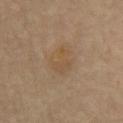Image and clinical context: A 15 mm close-up tile from a total-body photography series done for melanoma screening. Captured under cross-polarized illumination. Located on the front of the torso. The total-body-photography lesion software estimated a footprint of about 8.5 mm², a shape eccentricity near 0.8, and two-axis asymmetry of about 0.25. A male patient approximately 70 years of age.